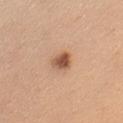biopsy_status: not biopsied; imaged during a skin examination
automated_metrics:
  area_mm2_approx: 5.0
  eccentricity: 0.65
  shape_asymmetry: 0.25
  border_irregularity_0_10: 2.0
  color_variation_0_10: 5.5
  peripheral_color_asymmetry: 2.0
  nevus_likeness_0_100: 100
  lesion_detection_confidence_0_100: 100
lighting: white-light
lesion_size:
  long_diameter_mm_approx: 3.0
patient:
  sex: male
  age_approx: 60
image:
  source: total-body photography crop
  field_of_view_mm: 15
site: left upper arm